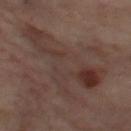This lesion was catalogued during total-body skin photography and was not selected for biopsy.
The lesion is on the left thigh.
About 13.5 mm across.
A region of skin cropped from a whole-body photographic capture, roughly 15 mm wide.
A female subject aged 53–57.
Imaged with cross-polarized lighting.